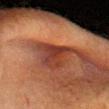Assessment: The lesion was tiled from a total-body skin photograph and was not biopsied. Acquisition and patient details: The subject is a female aged approximately 50. The total-body-photography lesion software estimated a footprint of about 6.5 mm² and an eccentricity of roughly 0.5. It also reported a mean CIELAB color near L≈31 a*≈24 b*≈26, roughly 10 lightness units darker than nearby skin, and a normalized border contrast of about 9.5. Cropped from a whole-body photographic skin survey; the tile spans about 15 mm. Approximately 3.5 mm at its widest. The tile uses cross-polarized illumination. The lesion is on the head or neck.